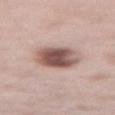| key | value |
|---|---|
| notes | catalogued during a skin exam; not biopsied |
| image | total-body-photography crop, ~15 mm field of view |
| automated lesion analysis | an area of roughly 13 mm², an eccentricity of roughly 0.35, and a shape-asymmetry score of about 0.1 (0 = symmetric); a classifier nevus-likeness of about 90/100 and a detector confidence of about 100 out of 100 that the crop contains a lesion |
| size | ≈4 mm |
| location | the abdomen |
| subject | male, aged 53–57 |
| lighting | white-light illumination |